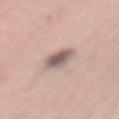lighting: white-light
image:
  source: total-body photography crop
  field_of_view_mm: 15
patient:
  sex: female
  age_approx: 60
site: mid back
lesion_size:
  long_diameter_mm_approx: 4.5